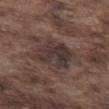{
  "biopsy_status": "not biopsied; imaged during a skin examination",
  "patient": {
    "sex": "male",
    "age_approx": 75
  },
  "site": "abdomen",
  "lighting": "white-light",
  "lesion_size": {
    "long_diameter_mm_approx": 4.5
  },
  "image": {
    "source": "total-body photography crop",
    "field_of_view_mm": 15
  }
}